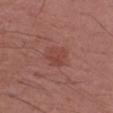The lesion was photographed on a routine skin check and not biopsied; there is no pathology result. Longest diameter approximately 2.5 mm. A male subject, roughly 70 years of age. Imaged with white-light lighting. Located on the right upper arm. A close-up tile cropped from a whole-body skin photograph, about 15 mm across.